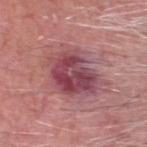This lesion was catalogued during total-body skin photography and was not selected for biopsy. About 6.5 mm across. A lesion tile, about 15 mm wide, cut from a 3D total-body photograph. On the head or neck. A male subject about 70 years old.